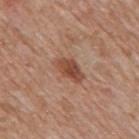follow-up=imaged on a skin check; not biopsied | subject=male, aged approximately 60 | site=the mid back | acquisition=~15 mm crop, total-body skin-cancer survey | lesion size=about 3.5 mm.